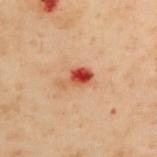Case summary:
– notes · catalogued during a skin exam; not biopsied
– lesion diameter · ≈3.5 mm
– subject · female, aged 58 to 62
– site · the upper back
– acquisition · ~15 mm crop, total-body skin-cancer survey